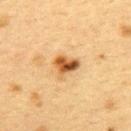This lesion was catalogued during total-body skin photography and was not selected for biopsy.
The subject is a female aged 38–42.
The total-body-photography lesion software estimated a mean CIELAB color near L≈48 a*≈19 b*≈37, about 14 CIELAB-L* units darker than the surrounding skin, and a normalized border contrast of about 10.
Cropped from a whole-body photographic skin survey; the tile spans about 15 mm.
Imaged with cross-polarized lighting.
From the upper back.